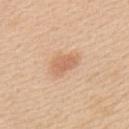Clinical impression: No biopsy was performed on this lesion — it was imaged during a full skin examination and was not determined to be concerning. Background: The total-body-photography lesion software estimated a lesion area of about 5.5 mm², an eccentricity of roughly 0.8, and a symmetry-axis asymmetry near 0.3. The analysis additionally found a lesion color around L≈64 a*≈21 b*≈34 in CIELAB, about 9 CIELAB-L* units darker than the surrounding skin, and a lesion-to-skin contrast of about 6 (normalized; higher = more distinct). It also reported a border-irregularity index near 2.5/10, a color-variation rating of about 1.5/10, and radial color variation of about 0.5. Located on the upper back. Longest diameter approximately 3.5 mm. The subject is a male aged around 60. Captured under white-light illumination. Cropped from a whole-body photographic skin survey; the tile spans about 15 mm.